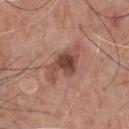biopsy status: total-body-photography surveillance lesion; no biopsy
subject: male, aged approximately 70
image source: ~15 mm tile from a whole-body skin photo
body site: the chest